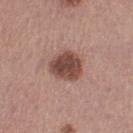Recorded during total-body skin imaging; not selected for excision or biopsy.
A lesion tile, about 15 mm wide, cut from a 3D total-body photograph.
The lesion is located on the right thigh.
Approximately 4 mm at its widest.
A female subject, aged approximately 50.
This is a white-light tile.